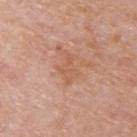| field | value |
|---|---|
| size | ≈4 mm |
| automated metrics | a lesion–skin lightness drop of about 7 and a normalized border contrast of about 5.5; a detector confidence of about 100 out of 100 that the crop contains a lesion |
| anatomic site | the upper back |
| patient | male, roughly 75 years of age |
| illumination | white-light illumination |
| image | ~15 mm tile from a whole-body skin photo |